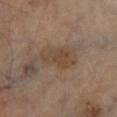A male subject aged approximately 70. A 15 mm crop from a total-body photograph taken for skin-cancer surveillance. Imaged with cross-polarized lighting. Automated image analysis of the tile measured an outline eccentricity of about 0.9 (0 = round, 1 = elongated) and a symmetry-axis asymmetry near 0.35. The analysis additionally found a border-irregularity index near 5/10, a color-variation rating of about 2.5/10, and peripheral color asymmetry of about 1. The lesion is on the right lower leg.